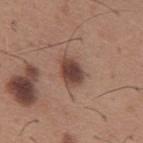Notes:
• biopsy status: catalogued during a skin exam; not biopsied